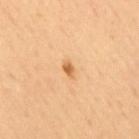- workup · imaged on a skin check; not biopsied
- illumination · cross-polarized illumination
- image · 15 mm crop, total-body photography
- lesion diameter · ≈2 mm
- subject · male, aged approximately 65
- image-analysis metrics · a footprint of about 2 mm², an eccentricity of roughly 0.8, and a shape-asymmetry score of about 0.35 (0 = symmetric); border irregularity of about 3 on a 0–10 scale, internal color variation of about 1.5 on a 0–10 scale, and radial color variation of about 0.5
- location · the back